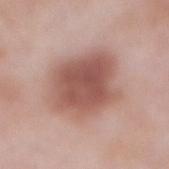{"biopsy_status": "not biopsied; imaged during a skin examination", "lesion_size": {"long_diameter_mm_approx": 7.5}, "lighting": "white-light", "image": {"source": "total-body photography crop", "field_of_view_mm": 15}, "patient": {"sex": "male", "age_approx": 55}, "site": "abdomen"}